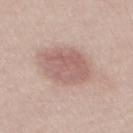  biopsy_status: not biopsied; imaged during a skin examination
  lesion_size:
    long_diameter_mm_approx: 5.0
  patient:
    sex: male
    age_approx: 40
  image:
    source: total-body photography crop
    field_of_view_mm: 15
  site: front of the torso